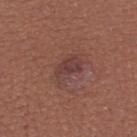Captured during whole-body skin photography for melanoma surveillance; the lesion was not biopsied. The lesion is on the right lower leg. The lesion's longest dimension is about 4 mm. Automated tile analysis of the lesion measured a lesion area of about 7.5 mm² and an outline eccentricity of about 0.8 (0 = round, 1 = elongated). It also reported an average lesion color of about L≈40 a*≈21 b*≈22 (CIELAB) and a normalized lesion–skin contrast near 6.5. The software also gave border irregularity of about 4 on a 0–10 scale and internal color variation of about 4 on a 0–10 scale. This is a white-light tile. A region of skin cropped from a whole-body photographic capture, roughly 15 mm wide. A female subject, in their mid-30s.